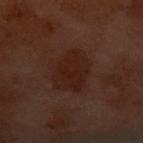| field | value |
|---|---|
| biopsy status | no biopsy performed (imaged during a skin exam) |
| subject | female, about 60 years old |
| tile lighting | cross-polarized illumination |
| lesion size | ~5 mm (longest diameter) |
| image-analysis metrics | a lesion color around L≈17 a*≈16 b*≈19 in CIELAB, a lesion–skin lightness drop of about 5, and a normalized border contrast of about 7; a border-irregularity rating of about 2/10 and peripheral color asymmetry of about 0.5 |
| imaging modality | ~15 mm tile from a whole-body skin photo |
| site | the front of the torso |